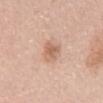Impression: Recorded during total-body skin imaging; not selected for excision or biopsy. Background: A male patient, about 55 years old. About 3 mm across. A 15 mm close-up tile from a total-body photography series done for melanoma screening. The tile uses white-light illumination. Automated tile analysis of the lesion measured a border-irregularity index near 3/10 and peripheral color asymmetry of about 0.5. The analysis additionally found a nevus-likeness score of about 40/100 and lesion-presence confidence of about 100/100. The lesion is on the abdomen.